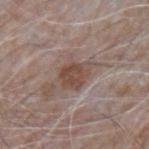{
  "biopsy_status": "not biopsied; imaged during a skin examination",
  "site": "left upper arm",
  "automated_metrics": {
    "area_mm2_approx": 8.5,
    "border_irregularity_0_10": 3.0,
    "nevus_likeness_0_100": 5,
    "lesion_detection_confidence_0_100": 100
  },
  "lighting": "white-light",
  "image": {
    "source": "total-body photography crop",
    "field_of_view_mm": 15
  },
  "patient": {
    "sex": "male",
    "age_approx": 65
  },
  "lesion_size": {
    "long_diameter_mm_approx": 4.5
  }
}